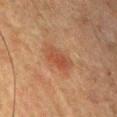* workup — catalogued during a skin exam; not biopsied
* image source — total-body-photography crop, ~15 mm field of view
* body site — the chest
* patient — male, about 50 years old